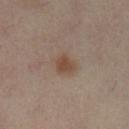Q: Was a biopsy performed?
A: no biopsy performed (imaged during a skin exam)
Q: Lesion location?
A: the right leg
Q: What kind of image is this?
A: total-body-photography crop, ~15 mm field of view
Q: How large is the lesion?
A: about 3 mm
Q: Who is the patient?
A: female, roughly 40 years of age